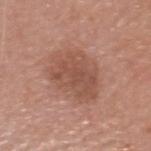Q: Was this lesion biopsied?
A: total-body-photography surveillance lesion; no biopsy
Q: What is the imaging modality?
A: ~15 mm tile from a whole-body skin photo
Q: What is the anatomic site?
A: the head or neck
Q: How was the tile lit?
A: white-light illumination
Q: How large is the lesion?
A: ≈6 mm
Q: What are the patient's age and sex?
A: male, roughly 60 years of age